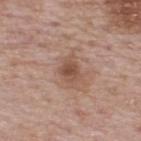Notes:
* follow-up · no biopsy performed (imaged during a skin exam)
* lesion size · ~3 mm (longest diameter)
* tile lighting · white-light
* image-analysis metrics · a lesion-to-skin contrast of about 7 (normalized; higher = more distinct)
* location · the upper back
* subject · male, aged around 65
* imaging modality · 15 mm crop, total-body photography